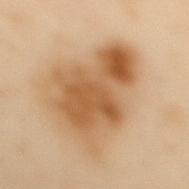notes: no biopsy performed (imaged during a skin exam)
body site: the upper back
TBP lesion metrics: a footprint of about 40 mm², an eccentricity of roughly 0.8, and a symmetry-axis asymmetry near 0.4; a nevus-likeness score of about 95/100 and lesion-presence confidence of about 100/100
tile lighting: cross-polarized illumination
diameter: ~10 mm (longest diameter)
subject: female, in their 60s
imaging modality: total-body-photography crop, ~15 mm field of view